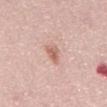Impression: The lesion was tiled from a total-body skin photograph and was not biopsied. Clinical summary: A male subject, about 50 years old. A roughly 15 mm field-of-view crop from a total-body skin photograph. The tile uses white-light illumination. On the abdomen. Longest diameter approximately 2.5 mm.